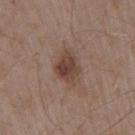{
  "biopsy_status": "not biopsied; imaged during a skin examination",
  "patient": {
    "sex": "male",
    "age_approx": 55
  },
  "site": "right upper arm",
  "lesion_size": {
    "long_diameter_mm_approx": 4.0
  },
  "image": {
    "source": "total-body photography crop",
    "field_of_view_mm": 15
  },
  "lighting": "white-light",
  "automated_metrics": {
    "area_mm2_approx": 8.5,
    "eccentricity": 0.7,
    "shape_asymmetry": 0.25,
    "cielab_L": 42,
    "cielab_a": 18,
    "cielab_b": 24,
    "border_irregularity_0_10": 2.5,
    "color_variation_0_10": 4.0,
    "peripheral_color_asymmetry": 1.0
  }
}